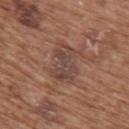Clinical impression:
No biopsy was performed on this lesion — it was imaged during a full skin examination and was not determined to be concerning.
Context:
Longest diameter approximately 4 mm. On the back. The tile uses white-light illumination. A female subject in their mid- to late 70s. A 15 mm close-up tile from a total-body photography series done for melanoma screening.